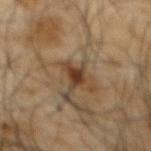<record>
<biopsy_status>not biopsied; imaged during a skin examination</biopsy_status>
<image>
  <source>total-body photography crop</source>
  <field_of_view_mm>15</field_of_view_mm>
</image>
<site>mid back</site>
<lesion_size>
  <long_diameter_mm_approx>3.0</long_diameter_mm_approx>
</lesion_size>
<automated_metrics>
  <eccentricity>0.8</eccentricity>
  <cielab_L>36</cielab_L>
  <cielab_a>17</cielab_a>
  <cielab_b>29</cielab_b>
  <vs_skin_darker_L>12.0</vs_skin_darker_L>
  <vs_skin_contrast_norm>10.0</vs_skin_contrast_norm>
  <border_irregularity_0_10>5.0</border_irregularity_0_10>
  <color_variation_0_10>2.5</color_variation_0_10>
  <peripheral_color_asymmetry>1.0</peripheral_color_asymmetry>
  <nevus_likeness_0_100>95</nevus_likeness_0_100>
  <lesion_detection_confidence_0_100>100</lesion_detection_confidence_0_100>
</automated_metrics>
<patient>
  <sex>male</sex>
  <age_approx>65</age_approx>
</patient>
<lighting>cross-polarized</lighting>
</record>